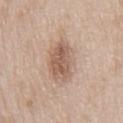The lesion was tiled from a total-body skin photograph and was not biopsied.
From the chest.
The patient is a male approximately 65 years of age.
This image is a 15 mm lesion crop taken from a total-body photograph.
This is a white-light tile.